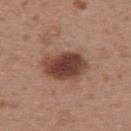<record>
  <biopsy_status>not biopsied; imaged during a skin examination</biopsy_status>
</record>